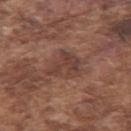| field | value |
|---|---|
| biopsy status | imaged on a skin check; not biopsied |
| site | the left upper arm |
| lighting | white-light |
| automated lesion analysis | a border-irregularity index near 7/10 and peripheral color asymmetry of about 0.5 |
| acquisition | total-body-photography crop, ~15 mm field of view |
| subject | male, aged approximately 75 |
| lesion diameter | about 4 mm |